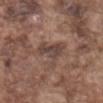Context:
Captured under white-light illumination. On the abdomen. This image is a 15 mm lesion crop taken from a total-body photograph. A male subject, in their mid-70s.